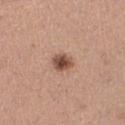Q: Is there a histopathology result?
A: no biopsy performed (imaged during a skin exam)
Q: Lesion size?
A: about 2.5 mm
Q: Illumination type?
A: white-light
Q: Where on the body is the lesion?
A: the left thigh
Q: Automated lesion metrics?
A: a lesion area of about 5 mm², an eccentricity of roughly 0.4, and two-axis asymmetry of about 0.15; a lesion color around L≈50 a*≈21 b*≈28 in CIELAB and about 15 CIELAB-L* units darker than the surrounding skin; a classifier nevus-likeness of about 95/100
Q: How was this image acquired?
A: ~15 mm tile from a whole-body skin photo
Q: Patient demographics?
A: female, in their 30s